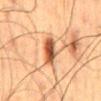biopsy status: imaged on a skin check; not biopsied | subject: male, aged 58 to 62 | site: the mid back | image source: ~15 mm crop, total-body skin-cancer survey | tile lighting: cross-polarized.